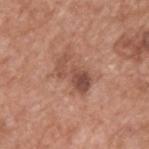| key | value |
|---|---|
| biopsy status | imaged on a skin check; not biopsied |
| acquisition | ~15 mm tile from a whole-body skin photo |
| automated metrics | a lesion area of about 8 mm² and an outline eccentricity of about 0.85 (0 = round, 1 = elongated); a normalized lesion–skin contrast near 7.5; a within-lesion color-variation index near 3.5/10 and radial color variation of about 1 |
| lighting | white-light |
| lesion size | about 4.5 mm |
| subject | male, aged 63–67 |
| body site | the upper back |